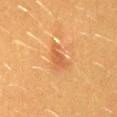A close-up tile cropped from a whole-body skin photograph, about 15 mm across.
The subject is a female approximately 40 years of age.
The lesion is on the lower back.